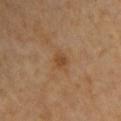Q: Was a biopsy performed?
A: total-body-photography surveillance lesion; no biopsy
Q: Who is the patient?
A: about 70 years old
Q: Automated lesion metrics?
A: an outline eccentricity of about 0.65 (0 = round, 1 = elongated); a nevus-likeness score of about 5/100
Q: What is the lesion's diameter?
A: about 2 mm
Q: What lighting was used for the tile?
A: cross-polarized illumination
Q: What is the anatomic site?
A: the front of the torso
Q: How was this image acquired?
A: 15 mm crop, total-body photography